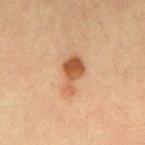subject = female, aged approximately 55
lesion diameter = ≈4.5 mm
lighting = cross-polarized illumination
location = the arm
image = 15 mm crop, total-body photography